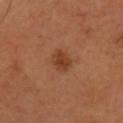Imaged during a routine full-body skin examination; the lesion was not biopsied and no histopathology is available.
The tile uses cross-polarized illumination.
Cropped from a whole-body photographic skin survey; the tile spans about 15 mm.
The lesion is located on the head or neck.
About 3 mm across.
The patient is a female approximately 60 years of age.